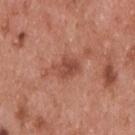Case summary:
* biopsy status: imaged on a skin check; not biopsied
* lesion size: ~3.5 mm (longest diameter)
* imaging modality: ~15 mm tile from a whole-body skin photo
* subject: male, about 55 years old
* location: the upper back
* illumination: white-light